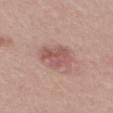follow-up: total-body-photography surveillance lesion; no biopsy | patient: male, in their mid-50s | size: ≈4.5 mm | illumination: white-light | anatomic site: the back | image-analysis metrics: an area of roughly 8.5 mm², an outline eccentricity of about 0.7 (0 = round, 1 = elongated), and a shape-asymmetry score of about 0.3 (0 = symmetric); a lesion color around L≈54 a*≈23 b*≈23 in CIELAB and a lesion–skin lightness drop of about 10; a nevus-likeness score of about 30/100 and a detector confidence of about 100 out of 100 that the crop contains a lesion | image source: total-body-photography crop, ~15 mm field of view.